A male patient, aged around 70 · a lesion tile, about 15 mm wide, cut from a 3D total-body photograph · on the upper back — 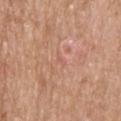Clinical summary:
This is a white-light tile. Approximately 2 mm at its widest.
Conclusion:
Histopathological examination showed a benign lesion: seborrheic keratosis.